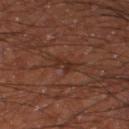biopsy_status: not biopsied; imaged during a skin examination